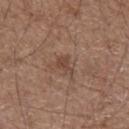notes = total-body-photography surveillance lesion; no biopsy | image-analysis metrics = an area of roughly 3 mm² and an eccentricity of roughly 0.75; a nevus-likeness score of about 0/100 | size = ≈2.5 mm | imaging modality = ~15 mm tile from a whole-body skin photo | subject = male, in their 50s | illumination = white-light illumination | location = the left forearm.